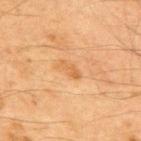{"biopsy_status": "not biopsied; imaged during a skin examination"}